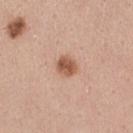The lesion is located on the left thigh. Longest diameter approximately 2.5 mm. The subject is a female aged approximately 45. A roughly 15 mm field-of-view crop from a total-body skin photograph.